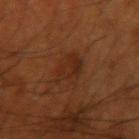  biopsy_status: not biopsied; imaged during a skin examination
  lesion_size:
    long_diameter_mm_approx: 4.0
  image:
    source: total-body photography crop
    field_of_view_mm: 15
  patient:
    sex: male
    age_approx: 70
  site: right upper arm
  automated_metrics:
    area_mm2_approx: 8.5
    eccentricity: 0.7
    border_irregularity_0_10: 3.0
    peripheral_color_asymmetry: 1.0
    lesion_detection_confidence_0_100: 100
  lighting: cross-polarized